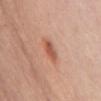This lesion was catalogued during total-body skin photography and was not selected for biopsy.
A female subject, roughly 70 years of age.
This is a white-light tile.
The lesion is on the front of the torso.
Approximately 3.5 mm at its widest.
A 15 mm close-up tile from a total-body photography series done for melanoma screening.